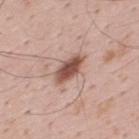Q: Is there a histopathology result?
A: total-body-photography surveillance lesion; no biopsy
Q: What kind of image is this?
A: 15 mm crop, total-body photography
Q: What lighting was used for the tile?
A: white-light illumination
Q: Lesion location?
A: the back
Q: What are the patient's age and sex?
A: male, about 35 years old
Q: How large is the lesion?
A: ~4 mm (longest diameter)